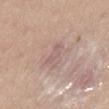follow-up: imaged on a skin check; not biopsied | location: the lower back | acquisition: ~15 mm crop, total-body skin-cancer survey | patient: male, aged 33–37 | lighting: white-light | lesion size: ~3 mm (longest diameter) | TBP lesion metrics: a lesion color around L≈59 a*≈18 b*≈20 in CIELAB; border irregularity of about 5 on a 0–10 scale and internal color variation of about 0 on a 0–10 scale; an automated nevus-likeness rating near 0 out of 100 and a lesion-detection confidence of about 95/100.